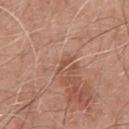  biopsy_status: not biopsied; imaged during a skin examination
  lesion_size:
    long_diameter_mm_approx: 2.5
  image:
    source: total-body photography crop
    field_of_view_mm: 15
  site: chest
  lighting: white-light
  patient:
    sex: male
    age_approx: 50
  automated_metrics:
    area_mm2_approx: 2.0
    eccentricity: 0.95
    shape_asymmetry: 0.45